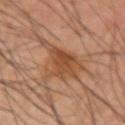{"biopsy_status": "not biopsied; imaged during a skin examination", "image": {"source": "total-body photography crop", "field_of_view_mm": 15}, "site": "arm", "lighting": "cross-polarized", "patient": {"sex": "male", "age_approx": 40}, "lesion_size": {"long_diameter_mm_approx": 7.5}, "automated_metrics": {"cielab_L": 48, "cielab_a": 22, "cielab_b": 33, "vs_skin_darker_L": 9.0, "vs_skin_contrast_norm": 7.5}}